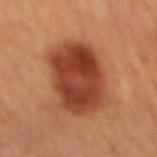The total-body-photography lesion software estimated a lesion–skin lightness drop of about 14 and a normalized lesion–skin contrast near 11. Cropped from a total-body skin-imaging series; the visible field is about 15 mm. Captured under cross-polarized illumination. The lesion is on the mid back. A male patient, about 70 years old.